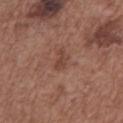image-analysis metrics — an area of roughly 2.5 mm², an outline eccentricity of about 0.9 (0 = round, 1 = elongated), and a symmetry-axis asymmetry near 0.4; a mean CIELAB color near L≈43 a*≈22 b*≈25, a lesion–skin lightness drop of about 8, and a normalized border contrast of about 6
image source — 15 mm crop, total-body photography
patient — male, about 75 years old
illumination — white-light illumination
size — about 2.5 mm
location — the chest